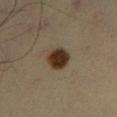{
  "biopsy_status": "not biopsied; imaged during a skin examination",
  "site": "right lower leg",
  "image": {
    "source": "total-body photography crop",
    "field_of_view_mm": 15
  },
  "lesion_size": {
    "long_diameter_mm_approx": 3.0
  },
  "patient": {
    "sex": "male",
    "age_approx": 35
  },
  "lighting": "cross-polarized"
}